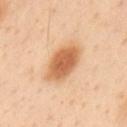biopsy_status: not biopsied; imaged during a skin examination
lesion_size:
  long_diameter_mm_approx: 5.5
patient:
  sex: male
  age_approx: 55
image:
  source: total-body photography crop
  field_of_view_mm: 15
site: upper back
lighting: cross-polarized